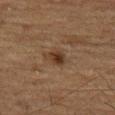A region of skin cropped from a whole-body photographic capture, roughly 15 mm wide.
A male patient, aged around 75.
The lesion-visualizer software estimated an area of roughly 4 mm², an eccentricity of roughly 0.6, and a symmetry-axis asymmetry near 0.2.
Imaged with cross-polarized lighting.
About 2.5 mm across.
The lesion is on the leg.